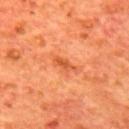Q: Is there a histopathology result?
A: no biopsy performed (imaged during a skin exam)
Q: Where on the body is the lesion?
A: the mid back
Q: How was the tile lit?
A: cross-polarized illumination
Q: How was this image acquired?
A: 15 mm crop, total-body photography
Q: Patient demographics?
A: male, aged 63–67